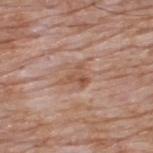Imaged during a routine full-body skin examination; the lesion was not biopsied and no histopathology is available. This is a white-light tile. The recorded lesion diameter is about 3.5 mm. The lesion is located on the upper back. A 15 mm close-up tile from a total-body photography series done for melanoma screening. A male subject, aged 58–62.